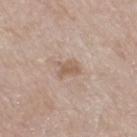Assessment:
No biopsy was performed on this lesion — it was imaged during a full skin examination and was not determined to be concerning.
Context:
The total-body-photography lesion software estimated an area of roughly 4 mm² and a shape eccentricity near 0.75. Approximately 2.5 mm at its widest. A 15 mm crop from a total-body photograph taken for skin-cancer surveillance. The patient is a female aged 73 to 77. The lesion is located on the right thigh.